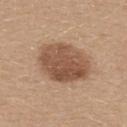follow-up: total-body-photography surveillance lesion; no biopsy
acquisition: ~15 mm crop, total-body skin-cancer survey
site: the upper back
size: about 6.5 mm
automated lesion analysis: a border-irregularity index near 1.5/10, a within-lesion color-variation index near 4.5/10, and a peripheral color-asymmetry measure near 2
subject: female, aged around 30